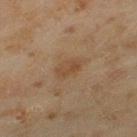Assessment: Imaged during a routine full-body skin examination; the lesion was not biopsied and no histopathology is available. Image and clinical context: A 15 mm crop from a total-body photograph taken for skin-cancer surveillance. Automated tile analysis of the lesion measured an area of roughly 5.5 mm², a shape eccentricity near 0.8, and a shape-asymmetry score of about 0.2 (0 = symmetric). The analysis additionally found an average lesion color of about L≈42 a*≈16 b*≈30 (CIELAB), roughly 6 lightness units darker than nearby skin, and a normalized border contrast of about 5.5. The analysis additionally found an automated nevus-likeness rating near 5 out of 100 and lesion-presence confidence of about 100/100. The lesion is on the left thigh. A female patient aged around 60.